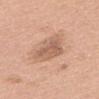Q: Was this lesion biopsied?
A: total-body-photography surveillance lesion; no biopsy
Q: What are the patient's age and sex?
A: female, approximately 60 years of age
Q: Automated lesion metrics?
A: roughly 10 lightness units darker than nearby skin and a normalized border contrast of about 6.5; a within-lesion color-variation index near 3.5/10 and peripheral color asymmetry of about 1
Q: How was the tile lit?
A: white-light illumination
Q: Where on the body is the lesion?
A: the upper back
Q: What is the imaging modality?
A: ~15 mm crop, total-body skin-cancer survey